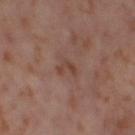notes = no biopsy performed (imaged during a skin exam) | patient = female, aged 53–57 | body site = the left thigh | acquisition = 15 mm crop, total-body photography.